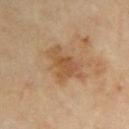<tbp_lesion>
  <patient>
    <sex>male</sex>
    <age_approx>65</age_approx>
  </patient>
  <automated_metrics>
    <area_mm2_approx>9.5</area_mm2_approx>
    <eccentricity>0.8</eccentricity>
    <shape_asymmetry>0.4</shape_asymmetry>
    <cielab_L>53</cielab_L>
    <cielab_a>20</cielab_a>
    <cielab_b>36</cielab_b>
    <vs_skin_darker_L>8.0</vs_skin_darker_L>
    <vs_skin_contrast_norm>6.5</vs_skin_contrast_norm>
    <border_irregularity_0_10>5.0</border_irregularity_0_10>
    <color_variation_0_10>3.5</color_variation_0_10>
    <peripheral_color_asymmetry>1.0</peripheral_color_asymmetry>
  </automated_metrics>
  <lesion_size>
    <long_diameter_mm_approx>4.5</long_diameter_mm_approx>
  </lesion_size>
  <site>left upper arm</site>
  <lighting>cross-polarized</lighting>
  <image>
    <source>total-body photography crop</source>
    <field_of_view_mm>15</field_of_view_mm>
  </image>
</tbp_lesion>